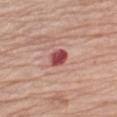Recorded during total-body skin imaging; not selected for excision or biopsy. Cropped from a whole-body photographic skin survey; the tile spans about 15 mm. The recorded lesion diameter is about 2.5 mm. A male subject, about 75 years old. Located on the left upper arm. The total-body-photography lesion software estimated a mean CIELAB color near L≈48 a*≈34 b*≈22, roughly 17 lightness units darker than nearby skin, and a normalized lesion–skin contrast near 11.5. It also reported border irregularity of about 1.5 on a 0–10 scale, internal color variation of about 3 on a 0–10 scale, and radial color variation of about 1.